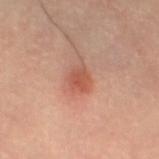Notes:
– notes: total-body-photography surveillance lesion; no biopsy
– patient: female, aged around 45
– body site: the left thigh
– acquisition: total-body-photography crop, ~15 mm field of view